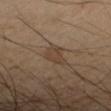Impression:
The lesion was photographed on a routine skin check and not biopsied; there is no pathology result.
Context:
The lesion's longest dimension is about 3.5 mm. The lesion is located on the right forearm. The subject is a female aged around 45. A 15 mm close-up tile from a total-body photography series done for melanoma screening. The tile uses cross-polarized illumination.